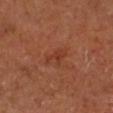follow-up = total-body-photography surveillance lesion; no biopsy
image = total-body-photography crop, ~15 mm field of view
location = the leg
patient = male, aged around 65